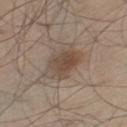Q: What are the patient's age and sex?
A: male, aged 58 to 62
Q: What kind of image is this?
A: 15 mm crop, total-body photography
Q: Illumination type?
A: white-light
Q: Lesion location?
A: the left thigh
Q: Automated lesion metrics?
A: a symmetry-axis asymmetry near 0.2; an average lesion color of about L≈48 a*≈13 b*≈25 (CIELAB), about 9 CIELAB-L* units darker than the surrounding skin, and a lesion-to-skin contrast of about 7 (normalized; higher = more distinct); border irregularity of about 2.5 on a 0–10 scale, internal color variation of about 5.5 on a 0–10 scale, and a peripheral color-asymmetry measure near 2; a classifier nevus-likeness of about 80/100 and a detector confidence of about 100 out of 100 that the crop contains a lesion
Q: Lesion size?
A: ~5 mm (longest diameter)